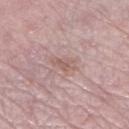subject = female, aged approximately 65 | location = the left thigh | image = ~15 mm crop, total-body skin-cancer survey.